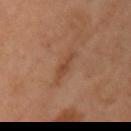The lesion was tiled from a total-body skin photograph and was not biopsied.